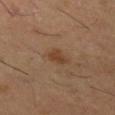Captured during whole-body skin photography for melanoma surveillance; the lesion was not biopsied.
The lesion is located on the left thigh.
The recorded lesion diameter is about 2.5 mm.
Captured under cross-polarized illumination.
The patient is a male about 60 years old.
The total-body-photography lesion software estimated a classifier nevus-likeness of about 80/100 and a detector confidence of about 100 out of 100 that the crop contains a lesion.
A close-up tile cropped from a whole-body skin photograph, about 15 mm across.